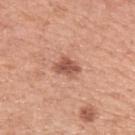Background: A female subject aged approximately 50. From the arm. Cropped from a whole-body photographic skin survey; the tile spans about 15 mm. The lesion's longest dimension is about 3 mm. This is a white-light tile.